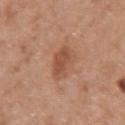follow-up: catalogued during a skin exam; not biopsied
image-analysis metrics: a footprint of about 7 mm², a shape eccentricity near 0.75, and a symmetry-axis asymmetry near 0.25; an automated nevus-likeness rating near 20 out of 100 and lesion-presence confidence of about 100/100
illumination: white-light illumination
diameter: ~3.5 mm (longest diameter)
location: the right forearm
subject: female, aged 58 to 62
image source: total-body-photography crop, ~15 mm field of view